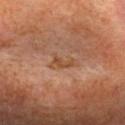follow-up: total-body-photography surveillance lesion; no biopsy
lesion diameter: ~3 mm (longest diameter)
anatomic site: the head or neck
automated lesion analysis: a lesion color around L≈41 a*≈19 b*≈31 in CIELAB, a lesion–skin lightness drop of about 6, and a normalized lesion–skin contrast near 6.5; a nevus-likeness score of about 0/100
lighting: cross-polarized illumination
patient: female, aged approximately 55
acquisition: 15 mm crop, total-body photography